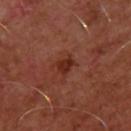Assessment: Captured during whole-body skin photography for melanoma surveillance; the lesion was not biopsied. Acquisition and patient details: The patient is a male about 50 years old. Automated tile analysis of the lesion measured an area of roughly 3.5 mm², an eccentricity of roughly 0.55, and a shape-asymmetry score of about 0.35 (0 = symmetric). And it measured a mean CIELAB color near L≈29 a*≈26 b*≈28, about 8 CIELAB-L* units darker than the surrounding skin, and a normalized border contrast of about 8.5. And it measured a color-variation rating of about 1/10 and peripheral color asymmetry of about 0.5. The analysis additionally found an automated nevus-likeness rating near 75 out of 100. The lesion is on the upper back. About 2 mm across. A lesion tile, about 15 mm wide, cut from a 3D total-body photograph. This is a cross-polarized tile.